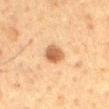This lesion was catalogued during total-body skin photography and was not selected for biopsy.
A 15 mm close-up tile from a total-body photography series done for melanoma screening.
The lesion is located on the mid back.
The subject is a male aged around 60.
Imaged with cross-polarized lighting.